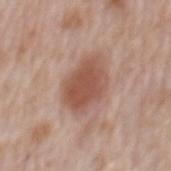follow-up = no biopsy performed (imaged during a skin exam).